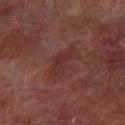notes=imaged on a skin check; not biopsied | body site=the left forearm | subject=male, roughly 65 years of age | illumination=cross-polarized illumination | lesion diameter=about 4 mm | image source=~15 mm crop, total-body skin-cancer survey.